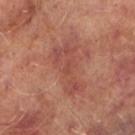biopsy status=no biopsy performed (imaged during a skin exam) | lesion diameter=≈5.5 mm | location=the left lower leg | illumination=cross-polarized illumination | subject=male, in their mid-60s | imaging modality=~15 mm tile from a whole-body skin photo.